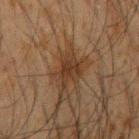Impression: This lesion was catalogued during total-body skin photography and was not selected for biopsy. Clinical summary: Automated image analysis of the tile measured a lesion color around L≈27 a*≈15 b*≈24 in CIELAB, about 7 CIELAB-L* units darker than the surrounding skin, and a lesion-to-skin contrast of about 7.5 (normalized; higher = more distinct). The software also gave a nevus-likeness score of about 5/100 and a detector confidence of about 95 out of 100 that the crop contains a lesion. From the right upper arm. A lesion tile, about 15 mm wide, cut from a 3D total-body photograph. A male patient, in their mid-30s.